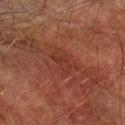- acquisition — 15 mm crop, total-body photography
- automated metrics — a shape eccentricity near 0.85 and a shape-asymmetry score of about 0.4 (0 = symmetric); border irregularity of about 4 on a 0–10 scale, a within-lesion color-variation index near 1.5/10, and peripheral color asymmetry of about 0.5
- location — the left forearm
- subject — male, aged approximately 75
- diameter — ≈3 mm
- lighting — cross-polarized illumination